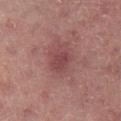{"biopsy_status": "not biopsied; imaged during a skin examination", "image": {"source": "total-body photography crop", "field_of_view_mm": 15}, "patient": {"sex": "male", "age_approx": 60}, "lighting": "cross-polarized", "site": "right lower leg"}